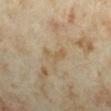Acquisition and patient details:
The recorded lesion diameter is about 3 mm. The subject is a female aged around 35. The total-body-photography lesion software estimated a lesion area of about 3.5 mm² and an outline eccentricity of about 0.9 (0 = round, 1 = elongated). The software also gave an average lesion color of about L≈54 a*≈12 b*≈33 (CIELAB) and about 6 CIELAB-L* units darker than the surrounding skin. And it measured a border-irregularity rating of about 6/10, a within-lesion color-variation index near 0/10, and a peripheral color-asymmetry measure near 0. And it measured a classifier nevus-likeness of about 0/100. The tile uses cross-polarized illumination. Cropped from a whole-body photographic skin survey; the tile spans about 15 mm. On the left forearm.